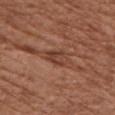<case>
<biopsy_status>not biopsied; imaged during a skin examination</biopsy_status>
<site>chest</site>
<lesion_size>
  <long_diameter_mm_approx>3.0</long_diameter_mm_approx>
</lesion_size>
<image>
  <source>total-body photography crop</source>
  <field_of_view_mm>15</field_of_view_mm>
</image>
<patient>
  <sex>female</sex>
  <age_approx>75</age_approx>
</patient>
<lighting>white-light</lighting>
</case>